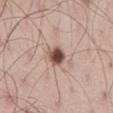Impression: The lesion was tiled from a total-body skin photograph and was not biopsied. Clinical summary: This image is a 15 mm lesion crop taken from a total-body photograph. A male patient about 55 years old. The tile uses white-light illumination. From the abdomen.